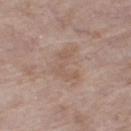Recorded during total-body skin imaging; not selected for excision or biopsy. Imaged with white-light lighting. A lesion tile, about 15 mm wide, cut from a 3D total-body photograph. From the right thigh. The total-body-photography lesion software estimated a lesion color around L≈56 a*≈17 b*≈26 in CIELAB and a normalized lesion–skin contrast near 4.5. The analysis additionally found a classifier nevus-likeness of about 0/100 and a lesion-detection confidence of about 100/100. The subject is a female roughly 75 years of age.